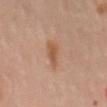Case summary:
- follow-up — total-body-photography surveillance lesion; no biopsy
- patient — female, about 60 years old
- body site — the mid back
- imaging modality — ~15 mm crop, total-body skin-cancer survey